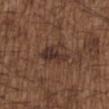{"biopsy_status": "not biopsied; imaged during a skin examination", "lighting": "white-light", "image": {"source": "total-body photography crop", "field_of_view_mm": 15}, "site": "chest", "patient": {"sex": "male", "age_approx": 50}, "lesion_size": {"long_diameter_mm_approx": 4.5}}